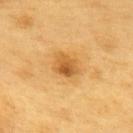Impression: The lesion was tiled from a total-body skin photograph and was not biopsied. Acquisition and patient details: A male subject aged 58–62. The tile uses cross-polarized illumination. The lesion's longest dimension is about 3 mm. On the upper back. A 15 mm close-up extracted from a 3D total-body photography capture.